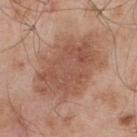Assessment:
No biopsy was performed on this lesion — it was imaged during a full skin examination and was not determined to be concerning.
Clinical summary:
Cropped from a total-body skin-imaging series; the visible field is about 15 mm. A male subject in their mid- to late 50s. Automated tile analysis of the lesion measured an area of roughly 35 mm². The analysis additionally found a border-irregularity index near 3.5/10. It also reported an automated nevus-likeness rating near 60 out of 100. Located on the upper back. Approximately 8.5 mm at its widest.